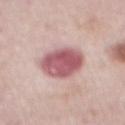Recorded during total-body skin imaging; not selected for excision or biopsy. A male patient, aged around 75. A lesion tile, about 15 mm wide, cut from a 3D total-body photograph. Captured under white-light illumination. The lesion is on the abdomen. Approximately 5 mm at its widest.